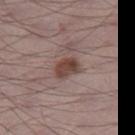subject: male, aged 68–72
lighting: white-light
image: total-body-photography crop, ~15 mm field of view
body site: the leg
diameter: about 3 mm
image-analysis metrics: a lesion area of about 5.5 mm² and an outline eccentricity of about 0.7 (0 = round, 1 = elongated); a lesion color around L≈43 a*≈18 b*≈22 in CIELAB, roughly 12 lightness units darker than nearby skin, and a normalized lesion–skin contrast near 9.5; a classifier nevus-likeness of about 90/100 and a detector confidence of about 100 out of 100 that the crop contains a lesion